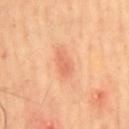Captured during whole-body skin photography for melanoma surveillance; the lesion was not biopsied.
A roughly 15 mm field-of-view crop from a total-body skin photograph.
Automated image analysis of the tile measured an average lesion color of about L≈65 a*≈29 b*≈37 (CIELAB) and a normalized border contrast of about 5.5. The analysis additionally found border irregularity of about 2.5 on a 0–10 scale, internal color variation of about 1.5 on a 0–10 scale, and peripheral color asymmetry of about 0.5. And it measured a classifier nevus-likeness of about 10/100 and a lesion-detection confidence of about 100/100.
A male patient about 65 years old.
From the chest.
Approximately 3 mm at its widest.